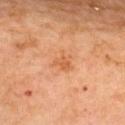lesion_size:
  long_diameter_mm_approx: 3.0
site: upper back
image:
  source: total-body photography crop
  field_of_view_mm: 15
patient:
  sex: female
  age_approx: 70
lighting: cross-polarized